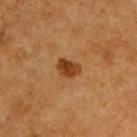Clinical impression:
Captured during whole-body skin photography for melanoma surveillance; the lesion was not biopsied.
Image and clinical context:
About 3 mm across. On the upper back. This is a cross-polarized tile. A roughly 15 mm field-of-view crop from a total-body skin photograph. A male patient about 75 years old.